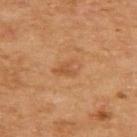Part of a total-body skin-imaging series; this lesion was reviewed on a skin check and was not flagged for biopsy. A lesion tile, about 15 mm wide, cut from a 3D total-body photograph. The recorded lesion diameter is about 3.5 mm. Imaged with cross-polarized lighting. The lesion is on the upper back. A female subject, aged 58–62. The lesion-visualizer software estimated a peripheral color-asymmetry measure near 1. It also reported a classifier nevus-likeness of about 0/100 and a lesion-detection confidence of about 100/100.